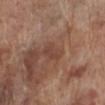Clinical impression: Imaged during a routine full-body skin examination; the lesion was not biopsied and no histopathology is available. Background: The subject is a male roughly 70 years of age. On the left lower leg. A region of skin cropped from a whole-body photographic capture, roughly 15 mm wide.